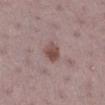Q: Is there a histopathology result?
A: catalogued during a skin exam; not biopsied
Q: What are the patient's age and sex?
A: female, aged around 40
Q: Lesion size?
A: ≈2.5 mm
Q: What is the anatomic site?
A: the right lower leg
Q: How was this image acquired?
A: ~15 mm crop, total-body skin-cancer survey
Q: How was the tile lit?
A: white-light